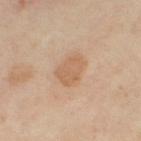follow-up: total-body-photography surveillance lesion; no biopsy
acquisition: ~15 mm tile from a whole-body skin photo
patient: female, aged 48 to 52
location: the right thigh